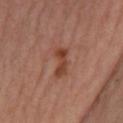<record>
  <biopsy_status>not biopsied; imaged during a skin examination</biopsy_status>
  <lighting>cross-polarized</lighting>
  <lesion_size>
    <long_diameter_mm_approx>4.0</long_diameter_mm_approx>
  </lesion_size>
  <image>
    <source>total-body photography crop</source>
    <field_of_view_mm>15</field_of_view_mm>
  </image>
  <automated_metrics>
    <area_mm2_approx>6.0</area_mm2_approx>
    <shape_asymmetry>0.35</shape_asymmetry>
    <nevus_likeness_0_100>0</nevus_likeness_0_100>
  </automated_metrics>
  <site>leg</site>
  <patient>
    <sex>female</sex>
    <age_approx>40</age_approx>
  </patient>
</record>